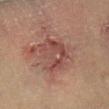– follow-up: no biopsy performed (imaged during a skin exam)
– illumination: cross-polarized
– image-analysis metrics: a lesion area of about 13 mm² and an eccentricity of roughly 0.65; an automated nevus-likeness rating near 0 out of 100 and a detector confidence of about 75 out of 100 that the crop contains a lesion
– body site: the right lower leg
– patient: male, in their mid- to late 60s
– diameter: ≈5 mm
– imaging modality: ~15 mm crop, total-body skin-cancer survey